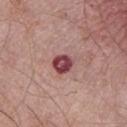Captured during whole-body skin photography for melanoma surveillance; the lesion was not biopsied. From the chest. This image is a 15 mm lesion crop taken from a total-body photograph. About 2.5 mm across. The patient is a male roughly 75 years of age.